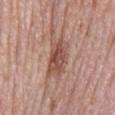biopsy_status: not biopsied; imaged during a skin examination
lighting: white-light
site: mid back
image:
  source: total-body photography crop
  field_of_view_mm: 15
patient:
  sex: male
  age_approx: 75
automated_metrics:
  area_mm2_approx: 12.0
  shape_asymmetry: 0.25
  nevus_likeness_0_100: 100
  lesion_detection_confidence_0_100: 100
lesion_size:
  long_diameter_mm_approx: 6.0